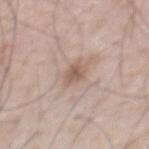Assessment: Part of a total-body skin-imaging series; this lesion was reviewed on a skin check and was not flagged for biopsy. Background: This image is a 15 mm lesion crop taken from a total-body photograph. The lesion is located on the mid back. The subject is a male about 55 years old. Approximately 2.5 mm at its widest.